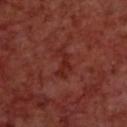notes — total-body-photography surveillance lesion; no biopsy | subject — male, roughly 70 years of age | image-analysis metrics — border irregularity of about 7 on a 0–10 scale, a within-lesion color-variation index near 1/10, and peripheral color asymmetry of about 0.5; a classifier nevus-likeness of about 0/100 and a lesion-detection confidence of about 95/100 | image source — ~15 mm crop, total-body skin-cancer survey | body site — the upper back | lighting — cross-polarized illumination.